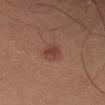Clinical impression: Part of a total-body skin-imaging series; this lesion was reviewed on a skin check and was not flagged for biopsy. Acquisition and patient details: On the right upper arm. Cropped from a whole-body photographic skin survey; the tile spans about 15 mm. A male subject in their mid-20s. The lesion-visualizer software estimated an area of roughly 5 mm², an eccentricity of roughly 0.65, and a symmetry-axis asymmetry near 0.15. The lesion's longest dimension is about 3 mm.